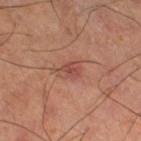Part of a total-body skin-imaging series; this lesion was reviewed on a skin check and was not flagged for biopsy.
The lesion-visualizer software estimated an average lesion color of about L≈46 a*≈24 b*≈26 (CIELAB), roughly 8 lightness units darker than nearby skin, and a lesion-to-skin contrast of about 6.5 (normalized; higher = more distinct). The software also gave a border-irregularity index near 2.5/10, internal color variation of about 3.5 on a 0–10 scale, and radial color variation of about 1. The analysis additionally found a nevus-likeness score of about 5/100 and lesion-presence confidence of about 100/100.
This is a cross-polarized tile.
A male patient approximately 60 years of age.
The lesion's longest dimension is about 2.5 mm.
The lesion is on the right thigh.
A 15 mm close-up tile from a total-body photography series done for melanoma screening.